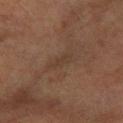This lesion was catalogued during total-body skin photography and was not selected for biopsy. A close-up tile cropped from a whole-body skin photograph, about 15 mm across. Located on the right forearm. The subject is a female aged 58–62.